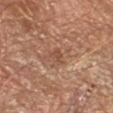Impression: No biopsy was performed on this lesion — it was imaged during a full skin examination and was not determined to be concerning. Image and clinical context: The tile uses cross-polarized illumination. The lesion's longest dimension is about 2.5 mm. Located on the arm. An algorithmic analysis of the crop reported a mean CIELAB color near L≈50 a*≈22 b*≈30, roughly 7 lightness units darker than nearby skin, and a normalized lesion–skin contrast near 5. And it measured a border-irregularity rating of about 4/10 and a within-lesion color-variation index near 2/10. It also reported a nevus-likeness score of about 0/100 and a detector confidence of about 100 out of 100 that the crop contains a lesion. A 15 mm close-up extracted from a 3D total-body photography capture.